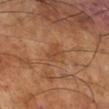Clinical summary: A region of skin cropped from a whole-body photographic capture, roughly 15 mm wide. Approximately 2.5 mm at its widest. From the right lower leg. Automated image analysis of the tile measured a symmetry-axis asymmetry near 0.3. And it measured lesion-presence confidence of about 100/100. The tile uses cross-polarized illumination. The subject is a male roughly 65 years of age.